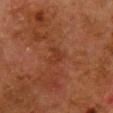Impression:
Recorded during total-body skin imaging; not selected for excision or biopsy.
Context:
From the chest. A female subject, in their 50s. A 15 mm crop from a total-body photograph taken for skin-cancer surveillance.